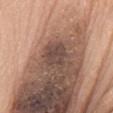biopsy_status: not biopsied; imaged during a skin examination
patient:
  sex: female
  age_approx: 60
lesion_size:
  long_diameter_mm_approx: 4.0
automated_metrics:
  eccentricity: 0.6
  shape_asymmetry: 0.3
  color_variation_0_10: 2.5
image:
  source: total-body photography crop
  field_of_view_mm: 15
site: chest
lighting: white-light